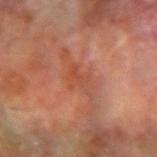biopsy status = total-body-photography surveillance lesion; no biopsy
illumination = cross-polarized illumination
size = ~3.5 mm (longest diameter)
patient = male, aged 68–72
location = the left forearm
automated lesion analysis = a footprint of about 4.5 mm² and an eccentricity of roughly 0.75; a mean CIELAB color near L≈48 a*≈28 b*≈34, roughly 6 lightness units darker than nearby skin, and a normalized lesion–skin contrast near 5.5; an automated nevus-likeness rating near 0 out of 100 and lesion-presence confidence of about 90/100
imaging modality = total-body-photography crop, ~15 mm field of view